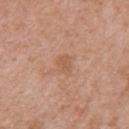Q: Is there a histopathology result?
A: catalogued during a skin exam; not biopsied
Q: Who is the patient?
A: male, aged around 60
Q: What is the imaging modality?
A: total-body-photography crop, ~15 mm field of view
Q: Where on the body is the lesion?
A: the right upper arm
Q: What is the lesion's diameter?
A: about 2.5 mm